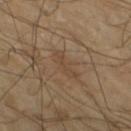Findings:
– follow-up — total-body-photography surveillance lesion; no biopsy
– site — the left lower leg
– acquisition — ~15 mm tile from a whole-body skin photo
– subject — male, aged 63–67